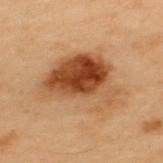Captured during whole-body skin photography for melanoma surveillance; the lesion was not biopsied. This image is a 15 mm lesion crop taken from a total-body photograph. About 8.5 mm across. The lesion is on the upper back. Captured under cross-polarized illumination. A male patient, about 55 years old.